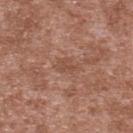Captured during whole-body skin photography for melanoma surveillance; the lesion was not biopsied. This is a white-light tile. From the upper back. The patient is a male aged around 45. The total-body-photography lesion software estimated border irregularity of about 3 on a 0–10 scale and internal color variation of about 1 on a 0–10 scale. A 15 mm crop from a total-body photograph taken for skin-cancer surveillance.